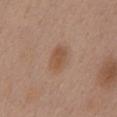Q: Was a biopsy performed?
A: no biopsy performed (imaged during a skin exam)
Q: What kind of image is this?
A: ~15 mm crop, total-body skin-cancer survey
Q: What is the anatomic site?
A: the mid back
Q: What are the patient's age and sex?
A: female, in their 50s